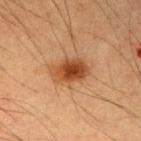Notes:
* biopsy status — total-body-photography surveillance lesion; no biopsy
* tile lighting — cross-polarized illumination
* site — the left upper arm
* automated metrics — a lesion color around L≈41 a*≈23 b*≈34 in CIELAB and a lesion-to-skin contrast of about 10 (normalized; higher = more distinct); a border-irregularity index near 2/10, a color-variation rating of about 5.5/10, and peripheral color asymmetry of about 1.5; a detector confidence of about 100 out of 100 that the crop contains a lesion
* imaging modality — total-body-photography crop, ~15 mm field of view
* lesion size — about 4.5 mm
* subject — male, approximately 55 years of age